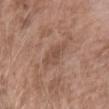{"biopsy_status": "not biopsied; imaged during a skin examination", "lesion_size": {"long_diameter_mm_approx": 3.5}, "lighting": "white-light", "image": {"source": "total-body photography crop", "field_of_view_mm": 15}, "site": "right forearm", "patient": {"sex": "female", "age_approx": 75}, "automated_metrics": {"cielab_L": 50, "cielab_a": 19, "cielab_b": 27, "vs_skin_darker_L": 7.0, "vs_skin_contrast_norm": 5.5, "border_irregularity_0_10": 3.0, "color_variation_0_10": 2.0}}